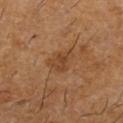follow-up = imaged on a skin check; not biopsied
image source = 15 mm crop, total-body photography
lesion size = about 3.5 mm
TBP lesion metrics = a border-irregularity rating of about 3.5/10 and internal color variation of about 2.5 on a 0–10 scale
patient = male, about 60 years old
tile lighting = cross-polarized
anatomic site = the left lower leg